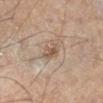Imaged during a routine full-body skin examination; the lesion was not biopsied and no histopathology is available. The recorded lesion diameter is about 2.5 mm. The subject is a male in their mid- to late 60s. The lesion is on the right lower leg. This image is a 15 mm lesion crop taken from a total-body photograph. Imaged with cross-polarized lighting.